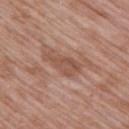This lesion was catalogued during total-body skin photography and was not selected for biopsy.
Cropped from a whole-body photographic skin survey; the tile spans about 15 mm.
A female subject, roughly 70 years of age.
Automated tile analysis of the lesion measured a footprint of about 7.5 mm², an outline eccentricity of about 0.9 (0 = round, 1 = elongated), and a shape-asymmetry score of about 0.35 (0 = symmetric). It also reported lesion-presence confidence of about 85/100.
Measured at roughly 4.5 mm in maximum diameter.
The lesion is located on the chest.